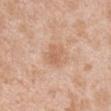{"biopsy_status": "not biopsied; imaged during a skin examination", "lighting": "white-light", "image": {"source": "total-body photography crop", "field_of_view_mm": 15}, "site": "left upper arm", "patient": {"sex": "male", "age_approx": 50}, "lesion_size": {"long_diameter_mm_approx": 3.0}, "automated_metrics": {"color_variation_0_10": 2.0, "peripheral_color_asymmetry": 0.5}}